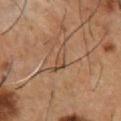The lesion was photographed on a routine skin check and not biopsied; there is no pathology result. A roughly 15 mm field-of-view crop from a total-body skin photograph. About 3.5 mm across. Captured under cross-polarized illumination. A male patient, aged approximately 55. The lesion is on the chest.